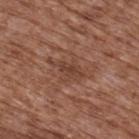Acquisition and patient details:
The lesion-visualizer software estimated a lesion area of about 4 mm², a shape eccentricity near 0.9, and two-axis asymmetry of about 0.35. It also reported a mean CIELAB color near L≈41 a*≈21 b*≈27, a lesion–skin lightness drop of about 8, and a normalized lesion–skin contrast near 6. The software also gave a border-irregularity rating of about 4.5/10, a color-variation rating of about 1.5/10, and peripheral color asymmetry of about 0.5. Located on the upper back. The tile uses white-light illumination. A 15 mm close-up tile from a total-body photography series done for melanoma screening. A male patient in their mid-70s. About 3.5 mm across.